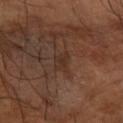Assessment:
This lesion was catalogued during total-body skin photography and was not selected for biopsy.
Background:
The recorded lesion diameter is about 2.5 mm. The lesion is located on the right forearm. Automated tile analysis of the lesion measured an average lesion color of about L≈30 a*≈17 b*≈24 (CIELAB), roughly 5 lightness units darker than nearby skin, and a lesion-to-skin contrast of about 5 (normalized; higher = more distinct). And it measured a border-irregularity index near 2.5/10, a color-variation rating of about 2/10, and peripheral color asymmetry of about 0.5. It also reported a detector confidence of about 80 out of 100 that the crop contains a lesion. A male patient, aged around 65. Cropped from a whole-body photographic skin survey; the tile spans about 15 mm. This is a cross-polarized tile.